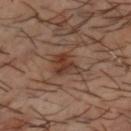Clinical impression:
No biopsy was performed on this lesion — it was imaged during a full skin examination and was not determined to be concerning.
Background:
The tile uses cross-polarized illumination. This image is a 15 mm lesion crop taken from a total-body photograph. The lesion's longest dimension is about 4.5 mm. A male patient, about 50 years old. On the head or neck.